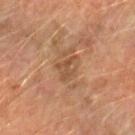Acquisition and patient details: Measured at roughly 3 mm in maximum diameter. A lesion tile, about 15 mm wide, cut from a 3D total-body photograph. Captured under cross-polarized illumination. A male subject, roughly 65 years of age. The lesion is located on the left forearm.